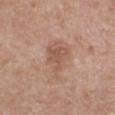This lesion was catalogued during total-body skin photography and was not selected for biopsy. The tile uses white-light illumination. Cropped from a total-body skin-imaging series; the visible field is about 15 mm. From the chest. A male patient approximately 80 years of age. The lesion's longest dimension is about 5.5 mm.